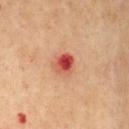Part of a total-body skin-imaging series; this lesion was reviewed on a skin check and was not flagged for biopsy. An algorithmic analysis of the crop reported a footprint of about 5 mm², a shape eccentricity near 0.5, and a shape-asymmetry score of about 0.2 (0 = symmetric). It also reported a nevus-likeness score of about 0/100 and a detector confidence of about 100 out of 100 that the crop contains a lesion. The lesion is located on the chest. Imaged with cross-polarized lighting. The subject is a male approximately 65 years of age. Approximately 2.5 mm at its widest. A lesion tile, about 15 mm wide, cut from a 3D total-body photograph.